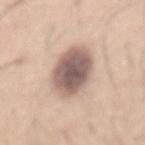No biopsy was performed on this lesion — it was imaged during a full skin examination and was not determined to be concerning.
About 6 mm across.
A male patient aged approximately 45.
A 15 mm close-up extracted from a 3D total-body photography capture.
Automated image analysis of the tile measured an average lesion color of about L≈58 a*≈16 b*≈22 (CIELAB) and roughly 17 lightness units darker than nearby skin. The software also gave a border-irregularity index near 1.5/10 and a within-lesion color-variation index near 5.5/10.
The tile uses white-light illumination.
The lesion is on the mid back.